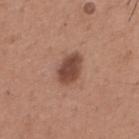{"biopsy_status": "not biopsied; imaged during a skin examination", "lighting": "white-light", "image": {"source": "total-body photography crop", "field_of_view_mm": 15}, "site": "back", "patient": {"sex": "male", "age_approx": 65}, "lesion_size": {"long_diameter_mm_approx": 4.0}}